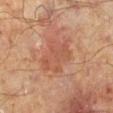Impression: The lesion was tiled from a total-body skin photograph and was not biopsied. Acquisition and patient details: A 15 mm close-up extracted from a 3D total-body photography capture. Imaged with cross-polarized lighting. About 5 mm across. A male patient aged approximately 65. Located on the leg.